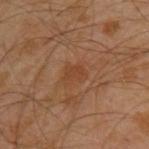{"biopsy_status": "not biopsied; imaged during a skin examination", "image": {"source": "total-body photography crop", "field_of_view_mm": 15}, "patient": {"sex": "male", "age_approx": 30}, "site": "left upper arm"}